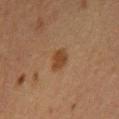Clinical impression: Recorded during total-body skin imaging; not selected for excision or biopsy. Background: A male patient approximately 65 years of age. The lesion is located on the chest. A roughly 15 mm field-of-view crop from a total-body skin photograph.